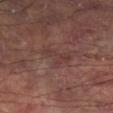{"biopsy_status": "not biopsied; imaged during a skin examination", "site": "right lower leg", "lighting": "cross-polarized", "lesion_size": {"long_diameter_mm_approx": 4.0}, "patient": {"sex": "male", "age_approx": 60}, "automated_metrics": {"eccentricity": 0.8, "shape_asymmetry": 0.55, "cielab_L": 36, "cielab_a": 19, "cielab_b": 20, "border_irregularity_0_10": 7.0, "color_variation_0_10": 2.5, "peripheral_color_asymmetry": 1.0}, "image": {"source": "total-body photography crop", "field_of_view_mm": 15}}